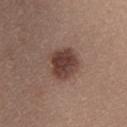follow-up: no biopsy performed (imaged during a skin exam).